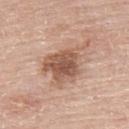notes: total-body-photography surveillance lesion; no biopsy
anatomic site: the left upper arm
illumination: white-light illumination
size: ≈6.5 mm
image source: ~15 mm tile from a whole-body skin photo
subject: male, aged 78 to 82